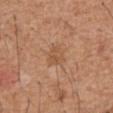Recorded during total-body skin imaging; not selected for excision or biopsy. The lesion is on the abdomen. The patient is a male aged 63 to 67. About 2.5 mm across. Cropped from a whole-body photographic skin survey; the tile spans about 15 mm.